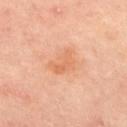The lesion was tiled from a total-body skin photograph and was not biopsied.
A region of skin cropped from a whole-body photographic capture, roughly 15 mm wide.
Located on the chest.
The lesion-visualizer software estimated a footprint of about 5.5 mm² and a symmetry-axis asymmetry near 0.4. The analysis additionally found a border-irregularity rating of about 4.5/10, a within-lesion color-variation index near 2.5/10, and a peripheral color-asymmetry measure near 1.
The tile uses cross-polarized illumination.
Longest diameter approximately 3 mm.
A female subject, aged 48–52.